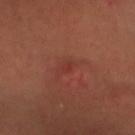workup: imaged on a skin check; not biopsied | patient: male, in their mid-50s | image source: total-body-photography crop, ~15 mm field of view | location: the head or neck | diameter: ~1.5 mm (longest diameter) | lighting: cross-polarized illumination.